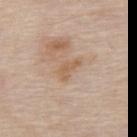Imaged during a routine full-body skin examination; the lesion was not biopsied and no histopathology is available.
About 3 mm across.
A region of skin cropped from a whole-body photographic capture, roughly 15 mm wide.
Imaged with white-light lighting.
Automated tile analysis of the lesion measured two-axis asymmetry of about 0.4. The analysis additionally found a lesion color around L≈59 a*≈17 b*≈32 in CIELAB, roughly 7 lightness units darker than nearby skin, and a lesion-to-skin contrast of about 6 (normalized; higher = more distinct). The software also gave a classifier nevus-likeness of about 0/100 and a lesion-detection confidence of about 100/100.
From the upper back.
The patient is a male aged approximately 85 or older.